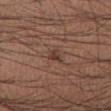biopsy status = total-body-photography surveillance lesion; no biopsy | acquisition = total-body-photography crop, ~15 mm field of view | subject = male, aged 38–42 | anatomic site = the right forearm.